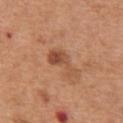Q: Was this lesion biopsied?
A: imaged on a skin check; not biopsied
Q: Lesion location?
A: the front of the torso
Q: Who is the patient?
A: male, aged 63 to 67
Q: Lesion size?
A: ≈4 mm
Q: What kind of image is this?
A: ~15 mm crop, total-body skin-cancer survey
Q: How was the tile lit?
A: white-light illumination